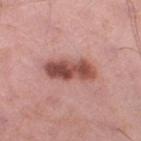Recorded during total-body skin imaging; not selected for excision or biopsy.
Located on the leg.
A roughly 15 mm field-of-view crop from a total-body skin photograph.
A male subject, aged 53–57.
Imaged with white-light lighting.
Longest diameter approximately 6 mm.